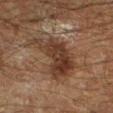Q: Lesion location?
A: the leg
Q: What is the imaging modality?
A: ~15 mm tile from a whole-body skin photo
Q: Automated lesion metrics?
A: a footprint of about 18 mm² and a shape eccentricity near 0.8; an average lesion color of about L≈27 a*≈14 b*≈22 (CIELAB) and a normalized lesion–skin contrast near 9.5; a nevus-likeness score of about 0/100 and lesion-presence confidence of about 100/100
Q: Who is the patient?
A: male, approximately 60 years of age
Q: What lighting was used for the tile?
A: cross-polarized illumination